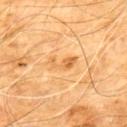<lesion>
  <biopsy_status>not biopsied; imaged during a skin examination</biopsy_status>
  <site>chest</site>
  <lesion_size>
    <long_diameter_mm_approx>3.5</long_diameter_mm_approx>
  </lesion_size>
  <lighting>cross-polarized</lighting>
  <image>
    <source>total-body photography crop</source>
    <field_of_view_mm>15</field_of_view_mm>
  </image>
  <patient>
    <sex>male</sex>
    <age_approx>60</age_approx>
  </patient>
</lesion>